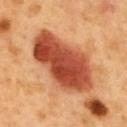Q: Is there a histopathology result?
A: total-body-photography surveillance lesion; no biopsy
Q: How large is the lesion?
A: ≈9 mm
Q: Who is the patient?
A: male, aged 48 to 52
Q: Where on the body is the lesion?
A: the mid back
Q: What kind of image is this?
A: ~15 mm tile from a whole-body skin photo
Q: Illumination type?
A: cross-polarized
Q: Automated lesion metrics?
A: a footprint of about 37 mm², an eccentricity of roughly 0.85, and two-axis asymmetry of about 0.2; an average lesion color of about L≈50 a*≈32 b*≈37 (CIELAB), a lesion–skin lightness drop of about 18, and a normalized border contrast of about 12; an automated nevus-likeness rating near 100 out of 100 and lesion-presence confidence of about 100/100